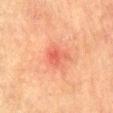Automated tile analysis of the lesion measured a lesion area of about 5 mm², an eccentricity of roughly 0.7, and a shape-asymmetry score of about 0.4 (0 = symmetric). The analysis additionally found an average lesion color of about L≈52 a*≈32 b*≈32 (CIELAB) and a normalized lesion–skin contrast near 5.5. And it measured border irregularity of about 4 on a 0–10 scale and radial color variation of about 1.
A male subject, aged around 75.
The lesion is on the lower back.
A 15 mm close-up tile from a total-body photography series done for melanoma screening.
Measured at roughly 3 mm in maximum diameter.
Captured under cross-polarized illumination.